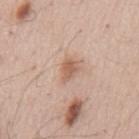Clinical impression:
Captured during whole-body skin photography for melanoma surveillance; the lesion was not biopsied.
Context:
A male subject, in their mid-50s. The total-body-photography lesion software estimated a footprint of about 4 mm², an outline eccentricity of about 0.75 (0 = round, 1 = elongated), and two-axis asymmetry of about 0.3. The analysis additionally found an average lesion color of about L≈60 a*≈20 b*≈30 (CIELAB) and a lesion–skin lightness drop of about 10. The lesion is located on the mid back. Captured under white-light illumination. This image is a 15 mm lesion crop taken from a total-body photograph.